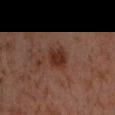Clinical impression: Captured during whole-body skin photography for melanoma surveillance; the lesion was not biopsied. Image and clinical context: The patient is a male aged around 30. A region of skin cropped from a whole-body photographic capture, roughly 15 mm wide. From the left forearm.